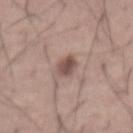Clinical impression: The lesion was photographed on a routine skin check and not biopsied; there is no pathology result. Background: Located on the abdomen. A male subject aged 38–42. A 15 mm crop from a total-body photograph taken for skin-cancer surveillance. This is a white-light tile. The lesion-visualizer software estimated an outline eccentricity of about 0.8 (0 = round, 1 = elongated). The analysis additionally found a mean CIELAB color near L≈51 a*≈17 b*≈22, about 12 CIELAB-L* units darker than the surrounding skin, and a normalized lesion–skin contrast near 8.5. It also reported a detector confidence of about 100 out of 100 that the crop contains a lesion.